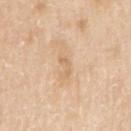| key | value |
|---|---|
| biopsy status | no biopsy performed (imaged during a skin exam) |
| lesion diameter | ~3 mm (longest diameter) |
| automated metrics | a lesion color around L≈68 a*≈18 b*≈37 in CIELAB, a lesion–skin lightness drop of about 7, and a normalized lesion–skin contrast near 5; a classifier nevus-likeness of about 0/100 and lesion-presence confidence of about 100/100 |
| patient | male, aged approximately 60 |
| location | the right upper arm |
| image | ~15 mm tile from a whole-body skin photo |
| illumination | white-light |